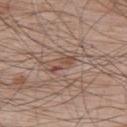Recorded during total-body skin imaging; not selected for excision or biopsy. A 15 mm close-up tile from a total-body photography series done for melanoma screening. A male subject roughly 65 years of age. The lesion is located on the upper back.